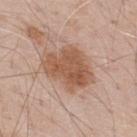Assessment: Part of a total-body skin-imaging series; this lesion was reviewed on a skin check and was not flagged for biopsy. Image and clinical context: Measured at roughly 6.5 mm in maximum diameter. The total-body-photography lesion software estimated an average lesion color of about L≈55 a*≈20 b*≈31 (CIELAB), roughly 11 lightness units darker than nearby skin, and a lesion-to-skin contrast of about 8.5 (normalized; higher = more distinct). The software also gave a border-irregularity index near 2.5/10 and internal color variation of about 3.5 on a 0–10 scale. A male patient aged approximately 70. Located on the upper back. Captured under white-light illumination. Cropped from a total-body skin-imaging series; the visible field is about 15 mm.